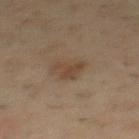{"biopsy_status": "not biopsied; imaged during a skin examination", "automated_metrics": {"area_mm2_approx": 4.5, "eccentricity": 0.45, "shape_asymmetry": 0.6, "border_irregularity_0_10": 7.0, "color_variation_0_10": 1.0, "peripheral_color_asymmetry": 0.5, "nevus_likeness_0_100": 10, "lesion_detection_confidence_0_100": 100}, "image": {"source": "total-body photography crop", "field_of_view_mm": 15}, "site": "mid back", "lesion_size": {"long_diameter_mm_approx": 3.0}, "patient": {"sex": "male", "age_approx": 55}, "lighting": "cross-polarized"}